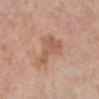Case summary:
* patient — female, about 70 years old
* tile lighting — white-light illumination
* image source — ~15 mm crop, total-body skin-cancer survey
* location — the left lower leg